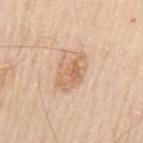• follow-up: total-body-photography surveillance lesion; no biopsy
• site: the left upper arm
• patient: male, about 70 years old
• diameter: about 4.5 mm
• image source: 15 mm crop, total-body photography
• illumination: white-light illumination